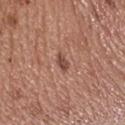No biopsy was performed on this lesion — it was imaged during a full skin examination and was not determined to be concerning.
A 15 mm close-up extracted from a 3D total-body photography capture.
This is a white-light tile.
The lesion is on the head or neck.
The lesion-visualizer software estimated an area of roughly 2.5 mm², a shape eccentricity near 0.85, and a shape-asymmetry score of about 0.3 (0 = symmetric). It also reported a mean CIELAB color near L≈47 a*≈23 b*≈26, roughly 11 lightness units darker than nearby skin, and a normalized border contrast of about 8. The analysis additionally found a border-irregularity rating of about 3/10, a within-lesion color-variation index near 0.5/10, and radial color variation of about 0.
About 2.5 mm across.
A male patient in their mid- to late 50s.